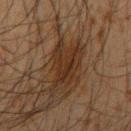The lesion was tiled from a total-body skin photograph and was not biopsied.
Cropped from a total-body skin-imaging series; the visible field is about 15 mm.
The patient is a male aged 33 to 37.
From the right upper arm.